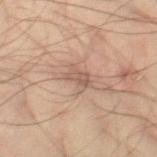Q: Is there a histopathology result?
A: no biopsy performed (imaged during a skin exam)
Q: Lesion location?
A: the right thigh
Q: What are the patient's age and sex?
A: male, aged around 35
Q: How was this image acquired?
A: total-body-photography crop, ~15 mm field of view